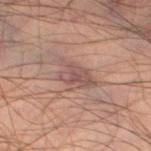  site: left thigh
  patient:
    sex: male
    age_approx: 45
  image:
    source: total-body photography crop
    field_of_view_mm: 15